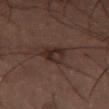Context: The lesion is located on the left thigh. A female patient aged 53 to 57. A 15 mm crop from a total-body photograph taken for skin-cancer surveillance. Imaged with cross-polarized lighting.